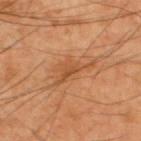{
  "biopsy_status": "not biopsied; imaged during a skin examination",
  "patient": {
    "sex": "male",
    "age_approx": 60
  },
  "lighting": "cross-polarized",
  "lesion_size": {
    "long_diameter_mm_approx": 5.0
  },
  "site": "upper back",
  "image": {
    "source": "total-body photography crop",
    "field_of_view_mm": 15
  }
}